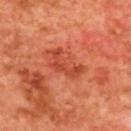follow-up: imaged on a skin check; not biopsied
acquisition: 15 mm crop, total-body photography
location: the back
patient: female, about 40 years old